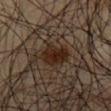* workup · catalogued during a skin exam; not biopsied